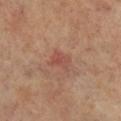Clinical impression:
Captured during whole-body skin photography for melanoma surveillance; the lesion was not biopsied.
Background:
This is a cross-polarized tile. A female patient, aged approximately 65. Located on the left leg. The total-body-photography lesion software estimated a border-irregularity index near 2/10, a color-variation rating of about 3/10, and radial color variation of about 1. A 15 mm crop from a total-body photograph taken for skin-cancer surveillance. Longest diameter approximately 2.5 mm.